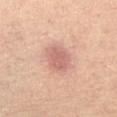Findings:
• follow-up · imaged on a skin check; not biopsied
• acquisition · ~15 mm tile from a whole-body skin photo
• lighting · cross-polarized illumination
• location · the leg
• diameter · about 4 mm
• automated lesion analysis · a footprint of about 9 mm² and an eccentricity of roughly 0.6; a mean CIELAB color near L≈60 a*≈23 b*≈26, a lesion–skin lightness drop of about 9, and a normalized border contrast of about 6; a classifier nevus-likeness of about 55/100 and lesion-presence confidence of about 100/100
• subject · female, aged approximately 45